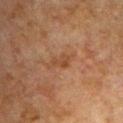biopsy status: imaged on a skin check; not biopsied
site: the chest
automated lesion analysis: an area of roughly 3.5 mm², an outline eccentricity of about 0.75 (0 = round, 1 = elongated), and two-axis asymmetry of about 0.35; a lesion color around L≈37 a*≈18 b*≈29 in CIELAB, a lesion–skin lightness drop of about 6, and a normalized lesion–skin contrast near 6; a color-variation rating of about 2/10 and a peripheral color-asymmetry measure near 0.5
image source: total-body-photography crop, ~15 mm field of view
lesion size: ~2.5 mm (longest diameter)
subject: male, in their 50s
lighting: cross-polarized